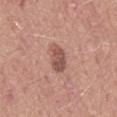Recorded during total-body skin imaging; not selected for excision or biopsy.
Automated image analysis of the tile measured an area of roughly 6.5 mm², an eccentricity of roughly 0.9, and a shape-asymmetry score of about 0.35 (0 = symmetric). The software also gave border irregularity of about 3 on a 0–10 scale and a color-variation rating of about 3.5/10.
A male subject, aged 48–52.
A 15 mm close-up tile from a total-body photography series done for melanoma screening.
The tile uses white-light illumination.
Approximately 4 mm at its widest.
From the mid back.